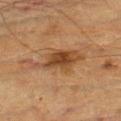site: left thigh
patient:
  sex: male
  age_approx: 85+
lighting: cross-polarized
image:
  source: total-body photography crop
  field_of_view_mm: 15